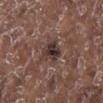Assessment:
No biopsy was performed on this lesion — it was imaged during a full skin examination and was not determined to be concerning.
Acquisition and patient details:
Cropped from a whole-body photographic skin survey; the tile spans about 15 mm. A male patient, about 70 years old. This is a white-light tile. From the right lower leg.